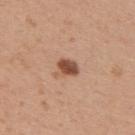Assessment: Recorded during total-body skin imaging; not selected for excision or biopsy. Image and clinical context: The lesion is located on the upper back. Longest diameter approximately 2.5 mm. The patient is a male in their 30s. The tile uses white-light illumination. Cropped from a total-body skin-imaging series; the visible field is about 15 mm. Automated image analysis of the tile measured a mean CIELAB color near L≈51 a*≈22 b*≈31, about 14 CIELAB-L* units darker than the surrounding skin, and a normalized border contrast of about 10. It also reported border irregularity of about 2 on a 0–10 scale and peripheral color asymmetry of about 1.